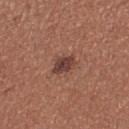Background: A close-up tile cropped from a whole-body skin photograph, about 15 mm across. The total-body-photography lesion software estimated an area of roughly 5 mm² and an outline eccentricity of about 0.75 (0 = round, 1 = elongated). The analysis additionally found a mean CIELAB color near L≈40 a*≈22 b*≈24, about 11 CIELAB-L* units darker than the surrounding skin, and a normalized lesion–skin contrast near 9.5. The software also gave a classifier nevus-likeness of about 65/100. The tile uses white-light illumination. The subject is a female roughly 35 years of age. Located on the leg.